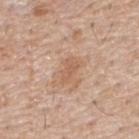Located on the back.
The patient is a male approximately 55 years of age.
This image is a 15 mm lesion crop taken from a total-body photograph.